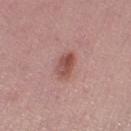The subject is a female aged 53 to 57.
A 15 mm crop from a total-body photograph taken for skin-cancer surveillance.
Automated tile analysis of the lesion measured an area of roughly 5.5 mm² and a shape-asymmetry score of about 0.25 (0 = symmetric). It also reported a border-irregularity index near 2.5/10, a within-lesion color-variation index near 5.5/10, and peripheral color asymmetry of about 2.
Located on the left lower leg.
Longest diameter approximately 3.5 mm.
The tile uses white-light illumination.